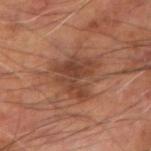Q: Was this lesion biopsied?
A: no biopsy performed (imaged during a skin exam)
Q: Illumination type?
A: cross-polarized
Q: Patient demographics?
A: male, roughly 60 years of age
Q: What is the anatomic site?
A: the left arm
Q: How large is the lesion?
A: ≈5 mm
Q: How was this image acquired?
A: 15 mm crop, total-body photography
Q: What did automated image analysis measure?
A: an area of roughly 13 mm² and a shape-asymmetry score of about 0.5 (0 = symmetric); a mean CIELAB color near L≈40 a*≈22 b*≈29, about 9 CIELAB-L* units darker than the surrounding skin, and a lesion-to-skin contrast of about 7 (normalized; higher = more distinct); a border-irregularity index near 6.5/10; an automated nevus-likeness rating near 0 out of 100